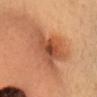Assessment: This lesion was catalogued during total-body skin photography and was not selected for biopsy. Image and clinical context: The lesion-visualizer software estimated an area of roughly 19 mm², a shape eccentricity near 0.75, and two-axis asymmetry of about 0.45. The software also gave a within-lesion color-variation index near 6.5/10 and peripheral color asymmetry of about 1.5. The software also gave a nevus-likeness score of about 15/100 and a detector confidence of about 100 out of 100 that the crop contains a lesion. The patient is a female aged 33–37. A 15 mm crop from a total-body photograph taken for skin-cancer surveillance. From the head or neck.